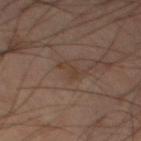No biopsy was performed on this lesion — it was imaged during a full skin examination and was not determined to be concerning. The patient is a male roughly 60 years of age. The tile uses cross-polarized illumination. The lesion is on the left thigh. The recorded lesion diameter is about 2.5 mm. An algorithmic analysis of the crop reported a lesion area of about 3 mm² and a symmetry-axis asymmetry near 0.55. The analysis additionally found a border-irregularity rating of about 5.5/10 and radial color variation of about 0. It also reported an automated nevus-likeness rating near 0 out of 100 and a detector confidence of about 100 out of 100 that the crop contains a lesion. A region of skin cropped from a whole-body photographic capture, roughly 15 mm wide.